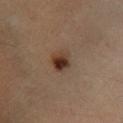- notes — total-body-photography surveillance lesion; no biopsy
- site — the left lower leg
- subject — male, in their 50s
- automated metrics — a footprint of about 6 mm² and a shape eccentricity near 0.5; a color-variation rating of about 9.5/10 and a peripheral color-asymmetry measure near 3; a classifier nevus-likeness of about 100/100 and lesion-presence confidence of about 100/100
- lighting — cross-polarized
- acquisition — ~15 mm crop, total-body skin-cancer survey